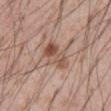location: the abdomen | subject: male, roughly 55 years of age | automated metrics: border irregularity of about 4 on a 0–10 scale, a color-variation rating of about 8/10, and a peripheral color-asymmetry measure near 2.5 | imaging modality: ~15 mm tile from a whole-body skin photo | illumination: white-light.